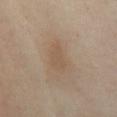Clinical impression: The lesion was tiled from a total-body skin photograph and was not biopsied. Image and clinical context: The patient is a female aged 53–57. Cropped from a whole-body photographic skin survey; the tile spans about 15 mm. The lesion is on the abdomen. An algorithmic analysis of the crop reported a within-lesion color-variation index near 2/10. The analysis additionally found a nevus-likeness score of about 0/100 and a lesion-detection confidence of about 100/100. About 4 mm across.